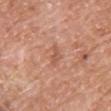- follow-up — no biopsy performed (imaged during a skin exam)
- anatomic site — the chest
- image — 15 mm crop, total-body photography
- subject — male, aged 73 to 77
- lesion diameter — ≈2.5 mm
- illumination — white-light